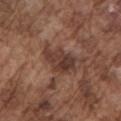Clinical impression: Imaged during a routine full-body skin examination; the lesion was not biopsied and no histopathology is available. Context: A male patient, aged 73 to 77. Located on the left upper arm. A 15 mm close-up extracted from a 3D total-body photography capture. The recorded lesion diameter is about 5 mm. The total-body-photography lesion software estimated a lesion color around L≈37 a*≈19 b*≈24 in CIELAB and about 10 CIELAB-L* units darker than the surrounding skin. The analysis additionally found a border-irregularity rating of about 3/10 and a within-lesion color-variation index near 5/10. It also reported a classifier nevus-likeness of about 10/100 and lesion-presence confidence of about 95/100.